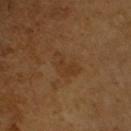Case summary:
• automated lesion analysis: a symmetry-axis asymmetry near 0.4; a border-irregularity index near 4.5/10, a within-lesion color-variation index near 2/10, and a peripheral color-asymmetry measure near 0.5
• image source: ~15 mm tile from a whole-body skin photo
• subject: male, aged 63–67
• size: ≈3.5 mm
• site: the right upper arm